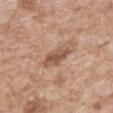Findings:
– lesion size — about 4 mm
– image source — ~15 mm crop, total-body skin-cancer survey
– illumination — white-light illumination
– patient — male, about 60 years old
– site — the chest
– TBP lesion metrics — a footprint of about 6.5 mm², a shape eccentricity near 0.85, and a shape-asymmetry score of about 0.25 (0 = symmetric); a mean CIELAB color near L≈54 a*≈20 b*≈28 and a normalized border contrast of about 7.5